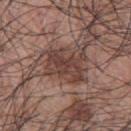notes — imaged on a skin check; not biopsied
acquisition — total-body-photography crop, ~15 mm field of view
patient — male, in their 70s
automated metrics — a lesion–skin lightness drop of about 10 and a normalized lesion–skin contrast near 8; a border-irregularity rating of about 6/10, internal color variation of about 3.5 on a 0–10 scale, and radial color variation of about 1.5
location — the upper back